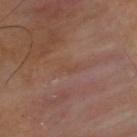Notes:
• workup — catalogued during a skin exam; not biopsied
• lesion size — about 3 mm
• tile lighting — cross-polarized
• subject — male, roughly 40 years of age
• location — the upper back
• imaging modality — total-body-photography crop, ~15 mm field of view